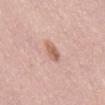Q: Was a biopsy performed?
A: total-body-photography surveillance lesion; no biopsy
Q: What is the lesion's diameter?
A: ≈2.5 mm
Q: How was this image acquired?
A: ~15 mm tile from a whole-body skin photo
Q: Lesion location?
A: the front of the torso
Q: Who is the patient?
A: female, aged approximately 65
Q: What did automated image analysis measure?
A: a lesion area of about 4 mm², an eccentricity of roughly 0.75, and a shape-asymmetry score of about 0.15 (0 = symmetric)
Q: How was the tile lit?
A: white-light